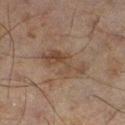| key | value |
|---|---|
| biopsy status | catalogued during a skin exam; not biopsied |
| automated lesion analysis | a lesion area of about 11 mm², a shape eccentricity near 0.9, and a shape-asymmetry score of about 0.45 (0 = symmetric); border irregularity of about 7 on a 0–10 scale, internal color variation of about 3.5 on a 0–10 scale, and radial color variation of about 1; a nevus-likeness score of about 0/100 and a lesion-detection confidence of about 100/100 |
| body site | the leg |
| lesion diameter | ≈5.5 mm |
| acquisition | 15 mm crop, total-body photography |
| subject | male, aged approximately 45 |
| tile lighting | cross-polarized |